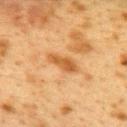Imaged during a routine full-body skin examination; the lesion was not biopsied and no histopathology is available.
The lesion's longest dimension is about 4 mm.
The subject is a female in their 40s.
An algorithmic analysis of the crop reported a lesion color around L≈49 a*≈21 b*≈39 in CIELAB, roughly 10 lightness units darker than nearby skin, and a normalized lesion–skin contrast near 7.5. It also reported a border-irregularity rating of about 3/10 and peripheral color asymmetry of about 1.
This is a cross-polarized tile.
A 15 mm crop from a total-body photograph taken for skin-cancer surveillance.
The lesion is located on the back.